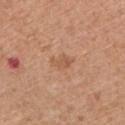Clinical impression: Recorded during total-body skin imaging; not selected for excision or biopsy. Clinical summary: A lesion tile, about 15 mm wide, cut from a 3D total-body photograph. This is a white-light tile. Located on the front of the torso. Approximately 2.5 mm at its widest. A female patient about 50 years old.